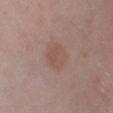image: total-body-photography crop, ~15 mm field of view
patient: male, approximately 50 years of age
automated metrics: an outline eccentricity of about 0.5 (0 = round, 1 = elongated) and a symmetry-axis asymmetry near 0.15; border irregularity of about 1.5 on a 0–10 scale and radial color variation of about 0.5
location: the left lower leg
tile lighting: white-light